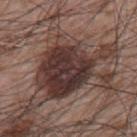Clinical impression: This lesion was catalogued during total-body skin photography and was not selected for biopsy. Clinical summary: The patient is a male approximately 65 years of age. Automated tile analysis of the lesion measured a mean CIELAB color near L≈34 a*≈17 b*≈19 and a normalized lesion–skin contrast near 12.5. The software also gave internal color variation of about 5.5 on a 0–10 scale and a peripheral color-asymmetry measure near 1.5. The analysis additionally found a nevus-likeness score of about 20/100 and lesion-presence confidence of about 95/100. Captured under white-light illumination. Longest diameter approximately 9.5 mm. A roughly 15 mm field-of-view crop from a total-body skin photograph. On the left upper arm.